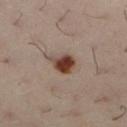Q: Was a biopsy performed?
A: no biopsy performed (imaged during a skin exam)
Q: Lesion location?
A: the left lower leg
Q: What are the patient's age and sex?
A: female, about 30 years old
Q: What kind of image is this?
A: 15 mm crop, total-body photography